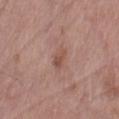{
  "biopsy_status": "not biopsied; imaged during a skin examination",
  "patient": {
    "sex": "female",
    "age_approx": 75
  },
  "image": {
    "source": "total-body photography crop",
    "field_of_view_mm": 15
  },
  "site": "right thigh",
  "lighting": "white-light"
}